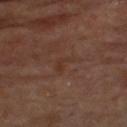Assessment: The lesion was photographed on a routine skin check and not biopsied; there is no pathology result. Context: A roughly 15 mm field-of-view crop from a total-body skin photograph. A male subject, aged approximately 65. The lesion's longest dimension is about 3 mm. Located on the chest.